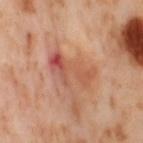No biopsy was performed on this lesion — it was imaged during a full skin examination and was not determined to be concerning.
From the leg.
A female subject, about 55 years old.
This image is a 15 mm lesion crop taken from a total-body photograph.
Automated tile analysis of the lesion measured a footprint of about 13 mm², a shape eccentricity near 0.85, and a shape-asymmetry score of about 0.35 (0 = symmetric). The software also gave a mean CIELAB color near L≈56 a*≈26 b*≈32, roughly 9 lightness units darker than nearby skin, and a normalized border contrast of about 6. The software also gave a border-irregularity rating of about 5.5/10 and radial color variation of about 3.5.
Approximately 5.5 mm at its widest.